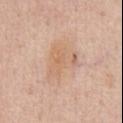Findings:
– TBP lesion metrics — an outline eccentricity of about 0.75 (0 = round, 1 = elongated) and a shape-asymmetry score of about 0.35 (0 = symmetric); a lesion color around L≈66 a*≈18 b*≈32 in CIELAB and a normalized lesion–skin contrast near 5
– illumination — white-light illumination
– image — ~15 mm crop, total-body skin-cancer survey
– subject — male, aged 48–52
– diameter — ≈6 mm
– location — the chest